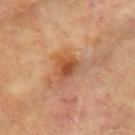  biopsy_status: not biopsied; imaged during a skin examination
  lighting: cross-polarized
  lesion_size:
    long_diameter_mm_approx: 3.0
  image:
    source: total-body photography crop
    field_of_view_mm: 15
  automated_metrics:
    lesion_detection_confidence_0_100: 100
  patient:
    sex: female
    age_approx: 75
  site: right upper arm